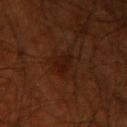No biopsy was performed on this lesion — it was imaged during a full skin examination and was not determined to be concerning.
From the right upper arm.
Longest diameter approximately 3 mm.
The subject is a male aged 68 to 72.
Cropped from a whole-body photographic skin survey; the tile spans about 15 mm.